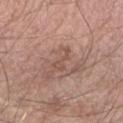The lesion was tiled from a total-body skin photograph and was not biopsied.
A male patient aged around 60.
A close-up tile cropped from a whole-body skin photograph, about 15 mm across.
Measured at roughly 3 mm in maximum diameter.
Located on the right forearm.